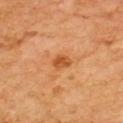{
  "biopsy_status": "not biopsied; imaged during a skin examination",
  "site": "upper back",
  "patient": {
    "sex": "male",
    "age_approx": 60
  },
  "lesion_size": {
    "long_diameter_mm_approx": 2.0
  },
  "lighting": "cross-polarized",
  "image": {
    "source": "total-body photography crop",
    "field_of_view_mm": 15
  }
}